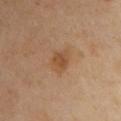This lesion was catalogued during total-body skin photography and was not selected for biopsy. The lesion is on the chest. A lesion tile, about 15 mm wide, cut from a 3D total-body photograph. A female patient roughly 40 years of age. Longest diameter approximately 3 mm. Captured under cross-polarized illumination.